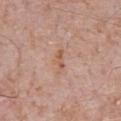biopsy_status: not biopsied; imaged during a skin examination
lesion_size:
  long_diameter_mm_approx: 3.0
image:
  source: total-body photography crop
  field_of_view_mm: 15
site: chest
lighting: white-light
patient:
  sex: male
  age_approx: 70